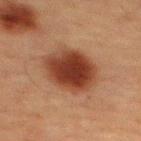The lesion was tiled from a total-body skin photograph and was not biopsied. Located on the upper back. The lesion's longest dimension is about 5.5 mm. The lesion-visualizer software estimated an automated nevus-likeness rating near 100 out of 100 and a lesion-detection confidence of about 100/100. A 15 mm crop from a total-body photograph taken for skin-cancer surveillance. The tile uses cross-polarized illumination. A male patient aged approximately 60.